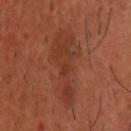The lesion was photographed on a routine skin check and not biopsied; there is no pathology result.
The total-body-photography lesion software estimated a lesion–skin lightness drop of about 6 and a lesion-to-skin contrast of about 5 (normalized; higher = more distinct).
The patient is a male about 40 years old.
A 15 mm close-up tile from a total-body photography series done for melanoma screening.
Longest diameter approximately 9.5 mm.
On the upper back.
The tile uses cross-polarized illumination.